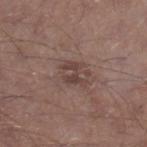biopsy status — no biopsy performed (imaged during a skin exam) | size — ≈3 mm | image — 15 mm crop, total-body photography | subject — male, aged 63–67 | body site — the right lower leg.